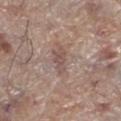Captured under white-light illumination.
About 3.5 mm across.
Automated tile analysis of the lesion measured a lesion area of about 4.5 mm² and a shape eccentricity near 0.9. It also reported an average lesion color of about L≈51 a*≈17 b*≈22 (CIELAB), a lesion–skin lightness drop of about 8, and a normalized border contrast of about 6. And it measured an automated nevus-likeness rating near 0 out of 100 and a detector confidence of about 95 out of 100 that the crop contains a lesion.
A 15 mm close-up tile from a total-body photography series done for melanoma screening.
A male patient aged around 70.
Located on the left lower leg.